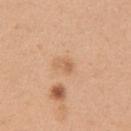<tbp_lesion>
  <biopsy_status>not biopsied; imaged during a skin examination</biopsy_status>
  <automated_metrics>
    <cielab_L>63</cielab_L>
    <cielab_a>21</cielab_a>
    <cielab_b>36</cielab_b>
    <border_irregularity_0_10>3.0</border_irregularity_0_10>
    <peripheral_color_asymmetry>0.5</peripheral_color_asymmetry>
  </automated_metrics>
  <image>
    <source>total-body photography crop</source>
    <field_of_view_mm>15</field_of_view_mm>
  </image>
  <patient>
    <sex>female</sex>
    <age_approx>30</age_approx>
  </patient>
  <site>right upper arm</site>
  <lesion_size>
    <long_diameter_mm_approx>2.5</long_diameter_mm_approx>
  </lesion_size>
  <lighting>white-light</lighting>
</tbp_lesion>